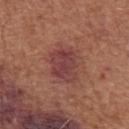The lesion was tiled from a total-body skin photograph and was not biopsied. Automated tile analysis of the lesion measured a footprint of about 10 mm² and an outline eccentricity of about 0.65 (0 = round, 1 = elongated). It also reported border irregularity of about 3 on a 0–10 scale, a within-lesion color-variation index near 3.5/10, and peripheral color asymmetry of about 1. The software also gave a classifier nevus-likeness of about 0/100 and a lesion-detection confidence of about 100/100. About 4 mm across. The tile uses white-light illumination. A close-up tile cropped from a whole-body skin photograph, about 15 mm across. From the arm. The subject is a male about 65 years old.